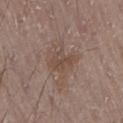workup: total-body-photography surveillance lesion; no biopsy | subject: male, aged around 20 | imaging modality: 15 mm crop, total-body photography | body site: the lower back | size: about 3.5 mm.